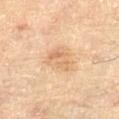Impression: This lesion was catalogued during total-body skin photography and was not selected for biopsy. Image and clinical context: About 4 mm across. The lesion is on the abdomen. This is a cross-polarized tile. A female subject aged 78 to 82. Automated image analysis of the tile measured a border-irregularity rating of about 3/10 and peripheral color asymmetry of about 1. The analysis additionally found a classifier nevus-likeness of about 0/100 and a detector confidence of about 100 out of 100 that the crop contains a lesion. A lesion tile, about 15 mm wide, cut from a 3D total-body photograph.